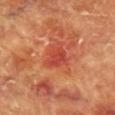Q: Is there a histopathology result?
A: catalogued during a skin exam; not biopsied
Q: Where on the body is the lesion?
A: the front of the torso
Q: Illumination type?
A: cross-polarized illumination
Q: What kind of image is this?
A: ~15 mm crop, total-body skin-cancer survey
Q: Who is the patient?
A: female, in their 80s